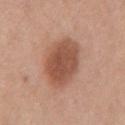{
  "biopsy_status": "not biopsied; imaged during a skin examination"
}